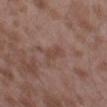No biopsy was performed on this lesion — it was imaged during a full skin examination and was not determined to be concerning. Longest diameter approximately 3 mm. Imaged with white-light lighting. A male patient, in their mid- to late 40s. The lesion-visualizer software estimated a color-variation rating of about 1.5/10 and peripheral color asymmetry of about 0.5. The lesion is located on the leg. A region of skin cropped from a whole-body photographic capture, roughly 15 mm wide.